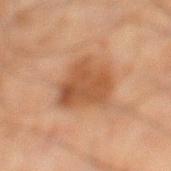No biopsy was performed on this lesion — it was imaged during a full skin examination and was not determined to be concerning. A lesion tile, about 15 mm wide, cut from a 3D total-body photograph. The total-body-photography lesion software estimated a shape eccentricity near 0.65 and a symmetry-axis asymmetry near 0.25. It also reported an average lesion color of about L≈43 a*≈19 b*≈30 (CIELAB). A male subject aged around 45. The lesion's longest dimension is about 6 mm. Captured under cross-polarized illumination. Located on the left lower leg.